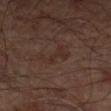Imaged during a routine full-body skin examination; the lesion was not biopsied and no histopathology is available. A male subject roughly 65 years of age. Captured under cross-polarized illumination. The lesion is on the left forearm. Cropped from a total-body skin-imaging series; the visible field is about 15 mm.